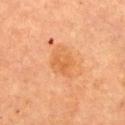Assessment:
Imaged during a routine full-body skin examination; the lesion was not biopsied and no histopathology is available.
Clinical summary:
A roughly 15 mm field-of-view crop from a total-body skin photograph. The lesion is located on the chest. A female patient, aged approximately 60. The total-body-photography lesion software estimated a normalized lesion–skin contrast near 5.5. Imaged with cross-polarized lighting. The lesion's longest dimension is about 4 mm.